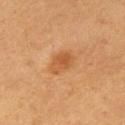The lesion was tiled from a total-body skin photograph and was not biopsied. The patient is a female approximately 55 years of age. A 15 mm crop from a total-body photograph taken for skin-cancer surveillance. The lesion is located on the right upper arm. Imaged with cross-polarized lighting. About 3.5 mm across. The total-body-photography lesion software estimated border irregularity of about 2 on a 0–10 scale, a color-variation rating of about 3/10, and a peripheral color-asymmetry measure near 1. And it measured a classifier nevus-likeness of about 65/100 and a lesion-detection confidence of about 100/100.